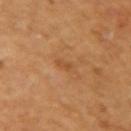Recorded during total-body skin imaging; not selected for excision or biopsy.
The lesion is on the arm.
A 15 mm crop from a total-body photograph taken for skin-cancer surveillance.
The patient is a female in their mid- to late 50s.